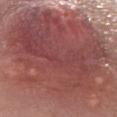workup = catalogued during a skin exam; not biopsied | anatomic site = the front of the torso | image-analysis metrics = an area of roughly 145 mm² and two-axis asymmetry of about 0.2 | subject = female, in their mid-40s | tile lighting = white-light | acquisition = 15 mm crop, total-body photography | lesion size = about 19 mm.